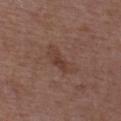Findings:
– workup — total-body-photography surveillance lesion; no biopsy
– location — the upper back
– lighting — white-light illumination
– subject — male, aged around 50
– lesion diameter — ≈4.5 mm
– acquisition — total-body-photography crop, ~15 mm field of view
– automated lesion analysis — an eccentricity of roughly 0.9 and a shape-asymmetry score of about 0.35 (0 = symmetric); a border-irregularity rating of about 4/10; a nevus-likeness score of about 5/100